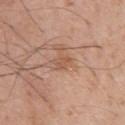Clinical impression:
Captured during whole-body skin photography for melanoma surveillance; the lesion was not biopsied.
Context:
A 15 mm crop from a total-body photograph taken for skin-cancer surveillance. From the arm. A male patient about 70 years old. Approximately 2.5 mm at its widest.